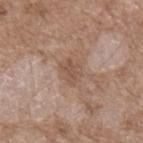{
  "biopsy_status": "not biopsied; imaged during a skin examination",
  "site": "left forearm",
  "patient": {
    "sex": "male",
    "age_approx": 50
  },
  "lesion_size": {
    "long_diameter_mm_approx": 3.0
  },
  "automated_metrics": {
    "nevus_likeness_0_100": 0,
    "lesion_detection_confidence_0_100": 100
  },
  "image": {
    "source": "total-body photography crop",
    "field_of_view_mm": 15
  }
}